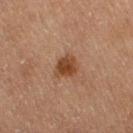Part of a total-body skin-imaging series; this lesion was reviewed on a skin check and was not flagged for biopsy. The subject is a female aged approximately 55. Imaged with cross-polarized lighting. From the right thigh. Approximately 2.5 mm at its widest. The lesion-visualizer software estimated an outline eccentricity of about 0.35 (0 = round, 1 = elongated) and a shape-asymmetry score of about 0.25 (0 = symmetric). The software also gave a border-irregularity rating of about 2/10, a color-variation rating of about 3.5/10, and radial color variation of about 1. And it measured a lesion-detection confidence of about 100/100. A roughly 15 mm field-of-view crop from a total-body skin photograph.